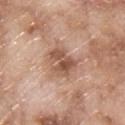  biopsy_status: not biopsied; imaged during a skin examination
  lighting: white-light
  lesion_size:
    long_diameter_mm_approx: 3.5
  automated_metrics:
    cielab_L: 53
    cielab_a: 21
    cielab_b: 29
    vs_skin_darker_L: 12.0
    vs_skin_contrast_norm: 8.0
    nevus_likeness_0_100: 0
    lesion_detection_confidence_0_100: 100
  patient:
    sex: male
    age_approx: 65
  image:
    source: total-body photography crop
    field_of_view_mm: 15
  site: upper back